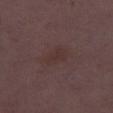Imaged during a routine full-body skin examination; the lesion was not biopsied and no histopathology is available. The subject is a female roughly 30 years of age. On the right thigh. Cropped from a whole-body photographic skin survey; the tile spans about 15 mm.